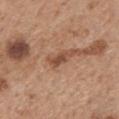Clinical impression:
The lesion was tiled from a total-body skin photograph and was not biopsied.
Clinical summary:
A 15 mm close-up tile from a total-body photography series done for melanoma screening. A male subject approximately 70 years of age. On the mid back.